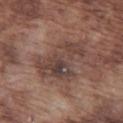{"biopsy_status": "not biopsied; imaged during a skin examination", "site": "left thigh", "image": {"source": "total-body photography crop", "field_of_view_mm": 15}, "patient": {"sex": "male", "age_approx": 75}}